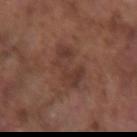Impression:
Captured during whole-body skin photography for melanoma surveillance; the lesion was not biopsied.
Clinical summary:
A 15 mm close-up tile from a total-body photography series done for melanoma screening. Imaged with white-light lighting. The lesion's longest dimension is about 4.5 mm. The lesion is on the right forearm. A male subject aged approximately 75.